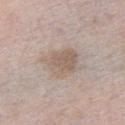workup = catalogued during a skin exam; not biopsied | acquisition = ~15 mm tile from a whole-body skin photo | lesion diameter = about 4.5 mm | automated metrics = a lesion color around L≈59 a*≈13 b*≈24 in CIELAB, roughly 9 lightness units darker than nearby skin, and a normalized lesion–skin contrast near 6; a border-irregularity index near 3/10, internal color variation of about 3.5 on a 0–10 scale, and a peripheral color-asymmetry measure near 1.5; an automated nevus-likeness rating near 15 out of 100 and a detector confidence of about 85 out of 100 that the crop contains a lesion | location = the chest | patient = female, aged around 40 | illumination = white-light illumination.